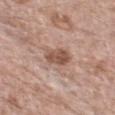{"lesion_size": {"long_diameter_mm_approx": 3.5}, "lighting": "white-light", "automated_metrics": {"eccentricity": 0.75}, "patient": {"sex": "female", "age_approx": 75}, "site": "chest", "image": {"source": "total-body photography crop", "field_of_view_mm": 15}}